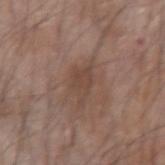• workup — imaged on a skin check; not biopsied
• subject — male, aged 68 to 72
• lesion size — ≈4.5 mm
• tile lighting — white-light illumination
• body site — the arm
• automated lesion analysis — a lesion area of about 7 mm² and two-axis asymmetry of about 0.4; a lesion color around L≈44 a*≈17 b*≈24 in CIELAB and a normalized lesion–skin contrast near 6; a border-irregularity rating of about 5/10 and a peripheral color-asymmetry measure near 0.5
• image — total-body-photography crop, ~15 mm field of view